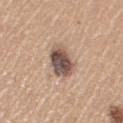Impression:
No biopsy was performed on this lesion — it was imaged during a full skin examination and was not determined to be concerning.
Clinical summary:
The lesion is located on the left upper arm. The subject is a female aged around 65. Imaged with white-light lighting. About 3.5 mm across. Cropped from a total-body skin-imaging series; the visible field is about 15 mm.